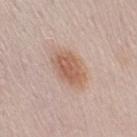Q: Is there a histopathology result?
A: total-body-photography surveillance lesion; no biopsy
Q: What kind of image is this?
A: ~15 mm crop, total-body skin-cancer survey
Q: Where on the body is the lesion?
A: the right upper arm
Q: Who is the patient?
A: female, aged 48 to 52